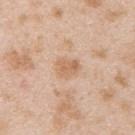Q: Is there a histopathology result?
A: total-body-photography surveillance lesion; no biopsy
Q: Lesion size?
A: ~3 mm (longest diameter)
Q: Where on the body is the lesion?
A: the upper back
Q: Who is the patient?
A: male, approximately 25 years of age
Q: Illumination type?
A: white-light illumination
Q: What kind of image is this?
A: total-body-photography crop, ~15 mm field of view